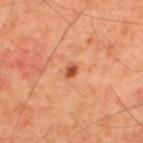notes: no biopsy performed (imaged during a skin exam)
imaging modality: 15 mm crop, total-body photography
anatomic site: the upper back
lesion size: about 1.5 mm
patient: male, aged around 60
TBP lesion metrics: a lesion area of about 2 mm², an outline eccentricity of about 0.65 (0 = round, 1 = elongated), and a symmetry-axis asymmetry near 0.2; an average lesion color of about L≈51 a*≈29 b*≈39 (CIELAB), a lesion–skin lightness drop of about 14, and a normalized lesion–skin contrast near 9; border irregularity of about 1.5 on a 0–10 scale, a within-lesion color-variation index near 1/10, and radial color variation of about 0.5; an automated nevus-likeness rating near 90 out of 100 and a lesion-detection confidence of about 100/100
lighting: cross-polarized illumination